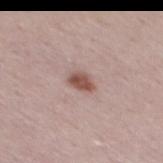A roughly 15 mm field-of-view crop from a total-body skin photograph. An algorithmic analysis of the crop reported a lesion area of about 4 mm², an outline eccentricity of about 0.7 (0 = round, 1 = elongated), and a symmetry-axis asymmetry near 0.2. The analysis additionally found about 13 CIELAB-L* units darker than the surrounding skin and a normalized border contrast of about 9.5. A male patient aged 28 to 32. Approximately 2.5 mm at its widest.